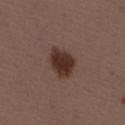The tile uses white-light illumination.
A 15 mm close-up tile from a total-body photography series done for melanoma screening.
Approximately 4 mm at its widest.
From the abdomen.
A female subject aged approximately 50.